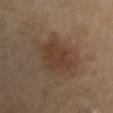From the right upper arm.
Captured under cross-polarized illumination.
A 15 mm crop from a total-body photograph taken for skin-cancer surveillance.